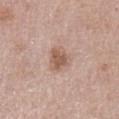biopsy status: imaged on a skin check; not biopsied
location: the left upper arm
lesion size: ~3.5 mm (longest diameter)
lighting: white-light
automated lesion analysis: a lesion area of about 6.5 mm² and a symmetry-axis asymmetry near 0.25; a border-irregularity index near 2.5/10, a color-variation rating of about 2.5/10, and radial color variation of about 1
image source: 15 mm crop, total-body photography
patient: female, aged 48 to 52